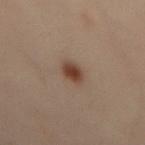biopsy status=catalogued during a skin exam; not biopsied | illumination=cross-polarized | TBP lesion metrics=a lesion-detection confidence of about 100/100 | patient=male, in their 50s | anatomic site=the back | size=≈2.5 mm | image=total-body-photography crop, ~15 mm field of view.